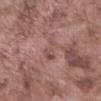{"biopsy_status": "not biopsied; imaged during a skin examination", "lighting": "white-light", "image": {"source": "total-body photography crop", "field_of_view_mm": 15}, "patient": {"sex": "male", "age_approx": 75}, "automated_metrics": {"border_irregularity_0_10": 5.0, "color_variation_0_10": 4.5, "peripheral_color_asymmetry": 1.5}, "site": "abdomen"}